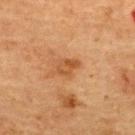Context: A female subject roughly 70 years of age. The recorded lesion diameter is about 2.5 mm. A close-up tile cropped from a whole-body skin photograph, about 15 mm across. On the back. Captured under cross-polarized illumination.